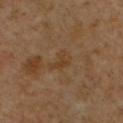Q: Was this lesion biopsied?
A: no biopsy performed (imaged during a skin exam)
Q: What kind of image is this?
A: ~15 mm crop, total-body skin-cancer survey
Q: What are the patient's age and sex?
A: female, in their mid-50s
Q: Where on the body is the lesion?
A: the right upper arm
Q: What is the lesion's diameter?
A: about 3.5 mm